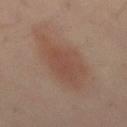notes = no biopsy performed (imaged during a skin exam) | TBP lesion metrics = a lesion color around L≈46 a*≈18 b*≈26 in CIELAB, about 7 CIELAB-L* units darker than the surrounding skin, and a normalized lesion–skin contrast near 6; a border-irregularity index near 4/10, a color-variation rating of about 3/10, and a peripheral color-asymmetry measure near 1; a classifier nevus-likeness of about 80/100 and lesion-presence confidence of about 100/100 | anatomic site = the mid back | image source = 15 mm crop, total-body photography | subject = male, approximately 40 years of age.